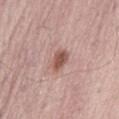Clinical impression:
The lesion was photographed on a routine skin check and not biopsied; there is no pathology result.
Clinical summary:
The recorded lesion diameter is about 3 mm. The lesion-visualizer software estimated a footprint of about 5 mm², a shape eccentricity near 0.8, and a symmetry-axis asymmetry near 0.2. It also reported an average lesion color of about L≈54 a*≈21 b*≈25 (CIELAB), about 13 CIELAB-L* units darker than the surrounding skin, and a lesion-to-skin contrast of about 9 (normalized; higher = more distinct). And it measured a border-irregularity index near 2/10 and radial color variation of about 0.5. On the abdomen. A 15 mm crop from a total-body photograph taken for skin-cancer surveillance. The tile uses white-light illumination. A male subject aged around 70.